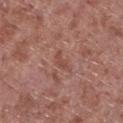biopsy status = catalogued during a skin exam; not biopsied
location = the left lower leg
imaging modality = total-body-photography crop, ~15 mm field of view
automated metrics = a lesion area of about 2.5 mm², an eccentricity of roughly 0.9, and a symmetry-axis asymmetry near 0.6; a nevus-likeness score of about 0/100
lighting = white-light
lesion diameter = ≈3 mm
patient = male, roughly 55 years of age